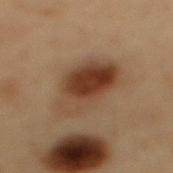Clinical impression:
Part of a total-body skin-imaging series; this lesion was reviewed on a skin check and was not flagged for biopsy.
Acquisition and patient details:
On the mid back. Imaged with cross-polarized lighting. The total-body-photography lesion software estimated a lesion area of about 21 mm², a shape eccentricity near 0.9, and a symmetry-axis asymmetry near 0.2. The software also gave a lesion color around L≈32 a*≈16 b*≈25 in CIELAB and a lesion–skin lightness drop of about 10. It also reported lesion-presence confidence of about 100/100. A close-up tile cropped from a whole-body skin photograph, about 15 mm across. Approximately 7.5 mm at its widest. A male subject, aged 53–57.